Located on the left upper arm; a 15 mm crop from a total-body photograph taken for skin-cancer surveillance; the patient is a male roughly 55 years of age.
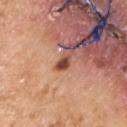| field | value |
|---|---|
| illumination | white-light illumination |
| size | ≈2 mm |
| TBP lesion metrics | an area of roughly 2 mm², a shape eccentricity near 0.8, and a symmetry-axis asymmetry near 0.3; an average lesion color of about L≈45 a*≈26 b*≈29 (CIELAB), roughly 18 lightness units darker than nearby skin, and a lesion-to-skin contrast of about 12.5 (normalized; higher = more distinct) |
| pathology | an atypical melanocytic neoplasm — a lesion of indeterminate malignant potential |